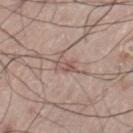Clinical impression: Imaged during a routine full-body skin examination; the lesion was not biopsied and no histopathology is available. Clinical summary: A male subject about 70 years old. A close-up tile cropped from a whole-body skin photograph, about 15 mm across. Longest diameter approximately 2.5 mm. Captured under white-light illumination. An algorithmic analysis of the crop reported a shape eccentricity near 0.85. And it measured an average lesion color of about L≈54 a*≈18 b*≈22 (CIELAB), a lesion–skin lightness drop of about 9, and a lesion-to-skin contrast of about 6 (normalized; higher = more distinct). The analysis additionally found border irregularity of about 4 on a 0–10 scale. The lesion is on the right leg.